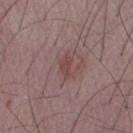<case>
<biopsy_status>not biopsied; imaged during a skin examination</biopsy_status>
<automated_metrics>
  <area_mm2_approx>3.0</area_mm2_approx>
  <eccentricity>0.8</eccentricity>
  <shape_asymmetry>0.45</shape_asymmetry>
  <cielab_L>44</cielab_L>
  <cielab_a>20</cielab_a>
  <cielab_b>19</cielab_b>
  <vs_skin_darker_L>7.0</vs_skin_darker_L>
  <vs_skin_contrast_norm>6.0</vs_skin_contrast_norm>
  <peripheral_color_asymmetry>0.0</peripheral_color_asymmetry>
  <nevus_likeness_0_100>0</nevus_likeness_0_100>
  <lesion_detection_confidence_0_100>100</lesion_detection_confidence_0_100>
</automated_metrics>
<lesion_size>
  <long_diameter_mm_approx>2.5</long_diameter_mm_approx>
</lesion_size>
<site>right upper arm</site>
<lighting>white-light</lighting>
<image>
  <source>total-body photography crop</source>
  <field_of_view_mm>15</field_of_view_mm>
</image>
<patient>
  <sex>male</sex>
  <age_approx>50</age_approx>
</patient>
</case>